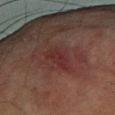This lesion was catalogued during total-body skin photography and was not selected for biopsy.
A male patient in their mid-70s.
From the arm.
A lesion tile, about 15 mm wide, cut from a 3D total-body photograph.
Automated tile analysis of the lesion measured a mean CIELAB color near L≈24 a*≈20 b*≈18, about 5 CIELAB-L* units darker than the surrounding skin, and a lesion-to-skin contrast of about 6 (normalized; higher = more distinct). And it measured a nevus-likeness score of about 0/100.